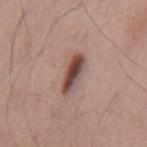Assessment:
No biopsy was performed on this lesion — it was imaged during a full skin examination and was not determined to be concerning.
Context:
Measured at roughly 4.5 mm in maximum diameter. Cropped from a total-body skin-imaging series; the visible field is about 15 mm. The subject is a male aged around 55. The lesion is on the mid back.